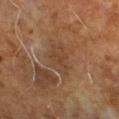This lesion was catalogued during total-body skin photography and was not selected for biopsy.
Captured under cross-polarized illumination.
The lesion is located on the right forearm.
The lesion's longest dimension is about 4.5 mm.
A region of skin cropped from a whole-body photographic capture, roughly 15 mm wide.
A male subject aged 68 to 72.